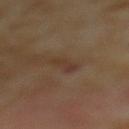Q: Was a biopsy performed?
A: total-body-photography surveillance lesion; no biopsy
Q: What lighting was used for the tile?
A: cross-polarized
Q: How large is the lesion?
A: about 3 mm
Q: How was this image acquired?
A: 15 mm crop, total-body photography
Q: What did automated image analysis measure?
A: two-axis asymmetry of about 0.35
Q: What is the anatomic site?
A: the mid back
Q: Who is the patient?
A: female, in their mid-50s